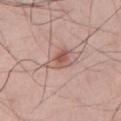Q: Was this lesion biopsied?
A: catalogued during a skin exam; not biopsied
Q: Who is the patient?
A: male, aged 48–52
Q: What did automated image analysis measure?
A: a mean CIELAB color near L≈56 a*≈22 b*≈25, about 10 CIELAB-L* units darker than the surrounding skin, and a normalized lesion–skin contrast near 7; a border-irregularity rating of about 4/10, internal color variation of about 6 on a 0–10 scale, and a peripheral color-asymmetry measure near 2
Q: What is the anatomic site?
A: the chest
Q: How was this image acquired?
A: 15 mm crop, total-body photography
Q: Lesion size?
A: about 3.5 mm
Q: How was the tile lit?
A: white-light